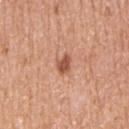Part of a total-body skin-imaging series; this lesion was reviewed on a skin check and was not flagged for biopsy. Approximately 2.5 mm at its widest. An algorithmic analysis of the crop reported a lesion area of about 3.5 mm² and an outline eccentricity of about 0.65 (0 = round, 1 = elongated). It also reported a lesion color around L≈54 a*≈26 b*≈33 in CIELAB, a lesion–skin lightness drop of about 13, and a normalized lesion–skin contrast near 8.5. The analysis additionally found a border-irregularity index near 2/10 and radial color variation of about 0.5. A female subject, aged 68 to 72. The lesion is on the right upper arm. Cropped from a whole-body photographic skin survey; the tile spans about 15 mm.